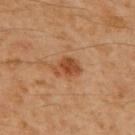Cropped from a whole-body photographic skin survey; the tile spans about 15 mm. A male patient, in their 60s. The lesion is located on the upper back.